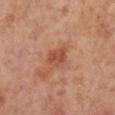{
  "biopsy_status": "not biopsied; imaged during a skin examination",
  "patient": {
    "sex": "female",
    "age_approx": 55
  },
  "image": {
    "source": "total-body photography crop",
    "field_of_view_mm": 15
  },
  "site": "right lower leg",
  "lesion_size": {
    "long_diameter_mm_approx": 3.0
  },
  "automated_metrics": {
    "area_mm2_approx": 5.0,
    "eccentricity": 0.7,
    "shape_asymmetry": 0.25,
    "border_irregularity_0_10": 2.0,
    "peripheral_color_asymmetry": 0.5
  },
  "lighting": "cross-polarized"
}